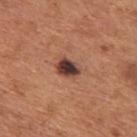Part of a total-body skin-imaging series; this lesion was reviewed on a skin check and was not flagged for biopsy. On the mid back. The lesion's longest dimension is about 3 mm. A lesion tile, about 15 mm wide, cut from a 3D total-body photograph. A male patient aged 63–67.